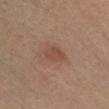Recorded during total-body skin imaging; not selected for excision or biopsy.
This is a cross-polarized tile.
The lesion is on the right lower leg.
A male patient aged 38 to 42.
A region of skin cropped from a whole-body photographic capture, roughly 15 mm wide.